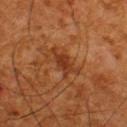<tbp_lesion>
  <biopsy_status>not biopsied; imaged during a skin examination</biopsy_status>
  <image>
    <source>total-body photography crop</source>
    <field_of_view_mm>15</field_of_view_mm>
  </image>
  <automated_metrics>
    <area_mm2_approx>6.0</area_mm2_approx>
    <eccentricity>0.9</eccentricity>
    <shape_asymmetry>0.4</shape_asymmetry>
  </automated_metrics>
  <lighting>cross-polarized</lighting>
  <lesion_size>
    <long_diameter_mm_approx>4.5</long_diameter_mm_approx>
  </lesion_size>
  <patient>
    <sex>male</sex>
    <age_approx>65</age_approx>
  </patient>
  <site>back</site>
</tbp_lesion>